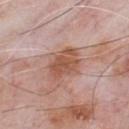Part of a total-body skin-imaging series; this lesion was reviewed on a skin check and was not flagged for biopsy. A male subject, aged around 60. A 15 mm close-up tile from a total-body photography series done for melanoma screening. Captured under white-light illumination. The lesion is located on the chest. The total-body-photography lesion software estimated a footprint of about 12 mm², a shape eccentricity near 0.7, and a shape-asymmetry score of about 0.25 (0 = symmetric). The software also gave border irregularity of about 3 on a 0–10 scale. The analysis additionally found lesion-presence confidence of about 100/100.